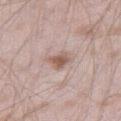Acquisition and patient details: On the right thigh. A 15 mm close-up extracted from a 3D total-body photography capture. The lesion-visualizer software estimated an average lesion color of about L≈57 a*≈18 b*≈24 (CIELAB), roughly 11 lightness units darker than nearby skin, and a normalized lesion–skin contrast near 8. And it measured a classifier nevus-likeness of about 65/100 and a detector confidence of about 100 out of 100 that the crop contains a lesion. Captured under white-light illumination. A male subject in their mid-40s. Longest diameter approximately 3 mm.